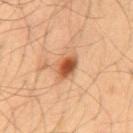Clinical impression:
Recorded during total-body skin imaging; not selected for excision or biopsy.
Acquisition and patient details:
A male subject, about 55 years old. The tile uses cross-polarized illumination. A 15 mm crop from a total-body photograph taken for skin-cancer surveillance. Longest diameter approximately 3.5 mm. Located on the mid back.